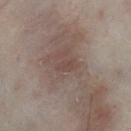Notes:
* biopsy status · no biopsy performed (imaged during a skin exam)
* subject · male, aged 48–52
* body site · the leg
* illumination · cross-polarized illumination
* imaging modality · ~15 mm tile from a whole-body skin photo